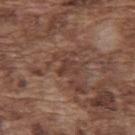Measured at roughly 2.5 mm in maximum diameter.
On the upper back.
Cropped from a whole-body photographic skin survey; the tile spans about 15 mm.
Imaged with white-light lighting.
The patient is a male aged 73 to 77.